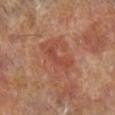workup = catalogued during a skin exam; not biopsied | body site = the left lower leg | subject = female, aged 73–77 | lesion size = ≈3.5 mm | tile lighting = cross-polarized illumination | acquisition = ~15 mm tile from a whole-body skin photo.